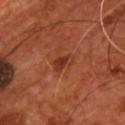Impression:
No biopsy was performed on this lesion — it was imaged during a full skin examination and was not determined to be concerning.